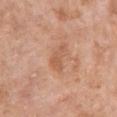Captured during whole-body skin photography for melanoma surveillance; the lesion was not biopsied. About 3.5 mm across. Automated image analysis of the tile measured a classifier nevus-likeness of about 0/100 and a lesion-detection confidence of about 100/100. The lesion is on the arm. Captured under white-light illumination. Cropped from a total-body skin-imaging series; the visible field is about 15 mm. A female subject aged approximately 70.